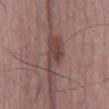Impression:
The lesion was photographed on a routine skin check and not biopsied; there is no pathology result.
Background:
The subject is a male aged 63 to 67. From the mid back. A 15 mm close-up extracted from a 3D total-body photography capture.